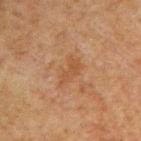Part of a total-body skin-imaging series; this lesion was reviewed on a skin check and was not flagged for biopsy.
This is a cross-polarized tile.
A male subject, aged approximately 60.
A close-up tile cropped from a whole-body skin photograph, about 15 mm across.
Longest diameter approximately 4 mm.
From the left arm.
Automated tile analysis of the lesion measured an average lesion color of about L≈38 a*≈17 b*≈29 (CIELAB), a lesion–skin lightness drop of about 5, and a normalized lesion–skin contrast near 5.5.